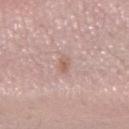| feature | finding |
|---|---|
| tile lighting | white-light illumination |
| lesion size | ~2.5 mm (longest diameter) |
| image | ~15 mm tile from a whole-body skin photo |
| patient | male, in their mid- to late 20s |
| anatomic site | the left forearm |